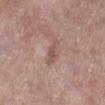Part of a total-body skin-imaging series; this lesion was reviewed on a skin check and was not flagged for biopsy. Approximately 3.5 mm at its widest. An algorithmic analysis of the crop reported an area of roughly 4 mm², an outline eccentricity of about 0.9 (0 = round, 1 = elongated), and two-axis asymmetry of about 0.3. The analysis additionally found border irregularity of about 4 on a 0–10 scale, a color-variation rating of about 1/10, and peripheral color asymmetry of about 0. Imaged with white-light lighting. The lesion is on the left lower leg. A 15 mm close-up extracted from a 3D total-body photography capture. A female subject approximately 65 years of age.